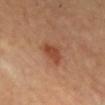The lesion was photographed on a routine skin check and not biopsied; there is no pathology result.
Cropped from a total-body skin-imaging series; the visible field is about 15 mm.
Located on the chest.
Approximately 3.5 mm at its widest.
The subject is a female in their 70s.
Automated tile analysis of the lesion measured a lesion area of about 6 mm² and two-axis asymmetry of about 0.35. The analysis additionally found border irregularity of about 3 on a 0–10 scale, a within-lesion color-variation index near 2/10, and a peripheral color-asymmetry measure near 1.